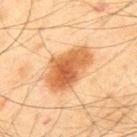This lesion was catalogued during total-body skin photography and was not selected for biopsy. From the back. A roughly 15 mm field-of-view crop from a total-body skin photograph. The patient is a male aged around 45. The tile uses cross-polarized illumination. Approximately 6.5 mm at its widest.